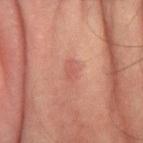A lesion tile, about 15 mm wide, cut from a 3D total-body photograph. The patient is a male aged around 75. This is a cross-polarized tile. The lesion is on the right forearm.